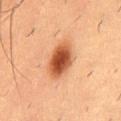The lesion was tiled from a total-body skin photograph and was not biopsied.
A close-up tile cropped from a whole-body skin photograph, about 15 mm across.
Measured at roughly 5 mm in maximum diameter.
A male patient, aged approximately 55.
Located on the lower back.
Imaged with cross-polarized lighting.